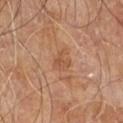Recorded during total-body skin imaging; not selected for excision or biopsy.
The lesion is on the leg.
The recorded lesion diameter is about 3 mm.
The total-body-photography lesion software estimated a footprint of about 4.5 mm² and a shape eccentricity near 0.55. And it measured about 6 CIELAB-L* units darker than the surrounding skin and a lesion-to-skin contrast of about 5 (normalized; higher = more distinct). The analysis additionally found a border-irregularity rating of about 4/10, a color-variation rating of about 2/10, and peripheral color asymmetry of about 1.
A 15 mm close-up tile from a total-body photography series done for melanoma screening.
A male subject aged approximately 60.
Captured under cross-polarized illumination.